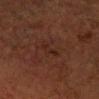Image and clinical context: The subject is a male roughly 65 years of age. The lesion is located on the head or neck. The tile uses cross-polarized illumination. Cropped from a whole-body photographic skin survey; the tile spans about 15 mm. Automated tile analysis of the lesion measured an area of roughly 4.5 mm² and a symmetry-axis asymmetry near 0.4. And it measured a normalized lesion–skin contrast near 4.5. It also reported a nevus-likeness score of about 5/100. Longest diameter approximately 3.5 mm.